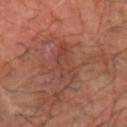Clinical impression: The lesion was photographed on a routine skin check and not biopsied; there is no pathology result.